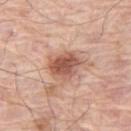{
  "biopsy_status": "not biopsied; imaged during a skin examination"
}